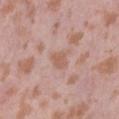The lesion was tiled from a total-body skin photograph and was not biopsied. The total-body-photography lesion software estimated a lesion color around L≈58 a*≈21 b*≈28 in CIELAB, roughly 7 lightness units darker than nearby skin, and a normalized lesion–skin contrast near 6. And it measured a border-irregularity index near 2.5/10, internal color variation of about 1.5 on a 0–10 scale, and peripheral color asymmetry of about 0.5. The software also gave a classifier nevus-likeness of about 0/100. From the abdomen. A female patient in their 40s. This image is a 15 mm lesion crop taken from a total-body photograph.